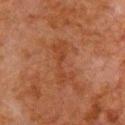| key | value |
|---|---|
| biopsy status | no biopsy performed (imaged during a skin exam) |
| body site | the chest |
| size | ~5.5 mm (longest diameter) |
| imaging modality | ~15 mm tile from a whole-body skin photo |
| patient | male, aged 78–82 |
| automated metrics | peripheral color asymmetry of about 0.5 |
| illumination | cross-polarized |